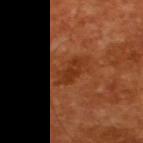Q: Is there a histopathology result?
A: no biopsy performed (imaged during a skin exam)
Q: Illumination type?
A: cross-polarized illumination
Q: Automated lesion metrics?
A: an automated nevus-likeness rating near 0 out of 100 and a lesion-detection confidence of about 100/100
Q: What are the patient's age and sex?
A: male, aged 63 to 67
Q: Lesion size?
A: about 4 mm
Q: What is the imaging modality?
A: 15 mm crop, total-body photography